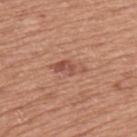Impression:
Part of a total-body skin-imaging series; this lesion was reviewed on a skin check and was not flagged for biopsy.
Context:
From the upper back. The subject is a male in their mid- to late 70s. This image is a 15 mm lesion crop taken from a total-body photograph. Captured under white-light illumination.